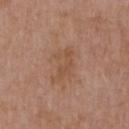Part of a total-body skin-imaging series; this lesion was reviewed on a skin check and was not flagged for biopsy. An algorithmic analysis of the crop reported a lesion color around L≈50 a*≈20 b*≈32 in CIELAB, about 6 CIELAB-L* units darker than the surrounding skin, and a normalized border contrast of about 5.5. On the right upper arm. Cropped from a whole-body photographic skin survey; the tile spans about 15 mm. The subject is a male aged around 50. Imaged with white-light lighting. About 4 mm across.